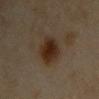Background: Cropped from a total-body skin-imaging series; the visible field is about 15 mm. The lesion is on the chest. The recorded lesion diameter is about 4 mm. A female subject, aged 33–37. Captured under cross-polarized illumination.